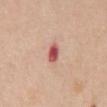This lesion was catalogued during total-body skin photography and was not selected for biopsy. The lesion is located on the chest. The lesion-visualizer software estimated an area of roughly 4 mm², an eccentricity of roughly 0.8, and a symmetry-axis asymmetry near 0.25. The subject is a male aged approximately 65. This image is a 15 mm lesion crop taken from a total-body photograph.